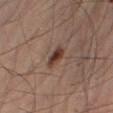{
  "biopsy_status": "not biopsied; imaged during a skin examination",
  "image": {
    "source": "total-body photography crop",
    "field_of_view_mm": 15
  },
  "site": "abdomen",
  "lighting": "cross-polarized",
  "lesion_size": {
    "long_diameter_mm_approx": 3.0
  },
  "patient": {
    "sex": "male",
    "age_approx": 50
  }
}